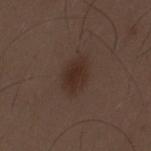notes: no biopsy performed (imaged during a skin exam)
lesion diameter: ≈4 mm
lighting: white-light illumination
image: total-body-photography crop, ~15 mm field of view
patient: male, aged 28 to 32
body site: the right upper arm